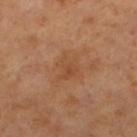Part of a total-body skin-imaging series; this lesion was reviewed on a skin check and was not flagged for biopsy. The subject is a female aged around 55. This image is a 15 mm lesion crop taken from a total-body photograph. The lesion is located on the left lower leg.